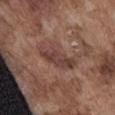Clinical summary: From the abdomen. The patient is a male aged around 75. A close-up tile cropped from a whole-body skin photograph, about 15 mm across. The tile uses white-light illumination.